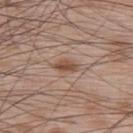The lesion was photographed on a routine skin check and not biopsied; there is no pathology result. A 15 mm crop from a total-body photograph taken for skin-cancer surveillance. The lesion-visualizer software estimated a lesion area of about 3.5 mm², an outline eccentricity of about 0.8 (0 = round, 1 = elongated), and a shape-asymmetry score of about 0.2 (0 = symmetric). Approximately 3 mm at its widest. On the back. A male patient, roughly 65 years of age.